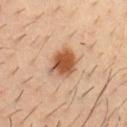Q: Was a biopsy performed?
A: catalogued during a skin exam; not biopsied